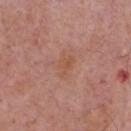Part of a total-body skin-imaging series; this lesion was reviewed on a skin check and was not flagged for biopsy. A 15 mm crop from a total-body photograph taken for skin-cancer surveillance. A male subject aged 73–77. The recorded lesion diameter is about 2.5 mm. Located on the chest. Imaged with white-light lighting.